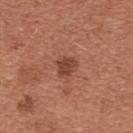Clinical impression:
Imaged during a routine full-body skin examination; the lesion was not biopsied and no histopathology is available.
Clinical summary:
This is a white-light tile. Located on the chest. This image is a 15 mm lesion crop taken from a total-body photograph. Automated image analysis of the tile measured an average lesion color of about L≈44 a*≈25 b*≈29 (CIELAB), about 10 CIELAB-L* units darker than the surrounding skin, and a normalized border contrast of about 7.5. A female subject aged around 35. The recorded lesion diameter is about 2.5 mm.